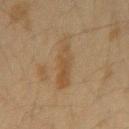site = the left forearm; subject = female, aged 38–42; tile lighting = cross-polarized; size = about 5 mm; acquisition = ~15 mm crop, total-body skin-cancer survey.